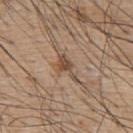workup = catalogued during a skin exam; not biopsied
acquisition = total-body-photography crop, ~15 mm field of view
automated lesion analysis = an average lesion color of about L≈49 a*≈16 b*≈28 (CIELAB); border irregularity of about 6 on a 0–10 scale, a color-variation rating of about 3.5/10, and a peripheral color-asymmetry measure near 1; an automated nevus-likeness rating near 5 out of 100 and a lesion-detection confidence of about 90/100
site = the upper back
subject = male, aged approximately 55